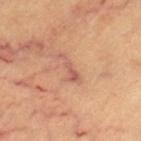biopsy status: catalogued during a skin exam; not biopsied | body site: the left thigh | lesion size: ~4 mm (longest diameter) | automated lesion analysis: an automated nevus-likeness rating near 0 out of 100 and lesion-presence confidence of about 85/100 | image: ~15 mm tile from a whole-body skin photo | subject: female, aged around 60 | illumination: cross-polarized illumination.